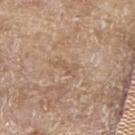Captured during whole-body skin photography for melanoma surveillance; the lesion was not biopsied.
A 15 mm close-up extracted from a 3D total-body photography capture.
An algorithmic analysis of the crop reported an eccentricity of roughly 0.85 and a symmetry-axis asymmetry near 0.55. And it measured about 6 CIELAB-L* units darker than the surrounding skin and a normalized border contrast of about 4.5. It also reported a border-irregularity rating of about 6/10, a within-lesion color-variation index near 0/10, and peripheral color asymmetry of about 0.
On the arm.
Measured at roughly 3 mm in maximum diameter.
A male subject, roughly 80 years of age.